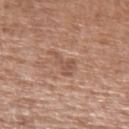This lesion was catalogued during total-body skin photography and was not selected for biopsy.
A male subject approximately 55 years of age.
The lesion's longest dimension is about 3.5 mm.
Imaged with white-light lighting.
From the left upper arm.
The total-body-photography lesion software estimated roughly 7 lightness units darker than nearby skin and a normalized lesion–skin contrast near 5.5. And it measured radial color variation of about 0.5.
A roughly 15 mm field-of-view crop from a total-body skin photograph.